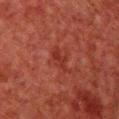<case>
<biopsy_status>not biopsied; imaged during a skin examination</biopsy_status>
<lighting>cross-polarized</lighting>
<automated_metrics>
  <area_mm2_approx>3.0</area_mm2_approx>
  <eccentricity>0.9</eccentricity>
  <shape_asymmetry>0.4</shape_asymmetry>
  <cielab_L>26</cielab_L>
  <cielab_a>26</cielab_a>
  <cielab_b>25</cielab_b>
  <vs_skin_darker_L>6.0</vs_skin_darker_L>
  <vs_skin_contrast_norm>6.0</vs_skin_contrast_norm>
</automated_metrics>
<image>
  <source>total-body photography crop</source>
  <field_of_view_mm>15</field_of_view_mm>
</image>
<patient>
  <sex>male</sex>
  <age_approx>60</age_approx>
</patient>
<site>chest</site>
</case>